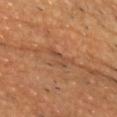| field | value |
|---|---|
| notes | total-body-photography surveillance lesion; no biopsy |
| patient | male, in their mid- to late 80s or older |
| imaging modality | total-body-photography crop, ~15 mm field of view |
| lesion size | ~3 mm (longest diameter) |
| tile lighting | cross-polarized illumination |
| image-analysis metrics | a lesion area of about 3.5 mm² and an eccentricity of roughly 0.9; roughly 6 lightness units darker than nearby skin and a lesion-to-skin contrast of about 5 (normalized; higher = more distinct); a border-irregularity index near 3.5/10 and peripheral color asymmetry of about 0 |
| site | the chest |